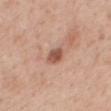| key | value |
|---|---|
| biopsy status | catalogued during a skin exam; not biopsied |
| site | the back |
| imaging modality | ~15 mm tile from a whole-body skin photo |
| lesion diameter | ~2.5 mm (longest diameter) |
| TBP lesion metrics | a nevus-likeness score of about 80/100 |
| patient | female, roughly 55 years of age |